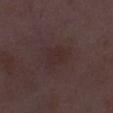Findings:
- imaging modality · total-body-photography crop, ~15 mm field of view
- site · the left thigh
- tile lighting · white-light
- subject · female, aged 28 to 32
- lesion size · about 3.5 mm